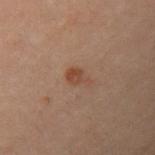* notes · imaged on a skin check; not biopsied
* subject · female, in their 30s
* size · about 2.5 mm
* automated metrics · a mean CIELAB color near L≈36 a*≈17 b*≈24; a classifier nevus-likeness of about 80/100 and a lesion-detection confidence of about 100/100
* acquisition · ~15 mm tile from a whole-body skin photo
* body site · the left upper arm